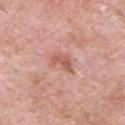The lesion was photographed on a routine skin check and not biopsied; there is no pathology result.
Imaged with white-light lighting.
A 15 mm close-up tile from a total-body photography series done for melanoma screening.
The total-body-photography lesion software estimated a classifier nevus-likeness of about 0/100 and a detector confidence of about 100 out of 100 that the crop contains a lesion.
Approximately 3 mm at its widest.
Located on the chest.
A male subject, approximately 55 years of age.